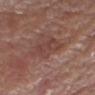Assessment: Imaged during a routine full-body skin examination; the lesion was not biopsied and no histopathology is available.